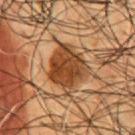Q: Is there a histopathology result?
A: total-body-photography surveillance lesion; no biopsy
Q: What did automated image analysis measure?
A: a classifier nevus-likeness of about 60/100 and lesion-presence confidence of about 95/100
Q: How was the tile lit?
A: cross-polarized
Q: What is the lesion's diameter?
A: ≈5 mm
Q: How was this image acquired?
A: ~15 mm crop, total-body skin-cancer survey
Q: Patient demographics?
A: male, in their mid-50s
Q: Where on the body is the lesion?
A: the chest